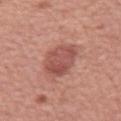Q: Was this lesion biopsied?
A: total-body-photography surveillance lesion; no biopsy